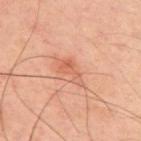biopsy_status: not biopsied; imaged during a skin examination
lighting: cross-polarized
patient:
  sex: male
  age_approx: 50
lesion_size:
  long_diameter_mm_approx: 4.0
site: upper back
image:
  source: total-body photography crop
  field_of_view_mm: 15